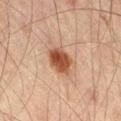biopsy_status: not biopsied; imaged during a skin examination
site: right thigh
image:
  source: total-body photography crop
  field_of_view_mm: 15
patient:
  sex: male
  age_approx: 45